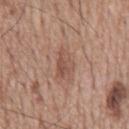The lesion was tiled from a total-body skin photograph and was not biopsied.
A male subject, about 60 years old.
The lesion is located on the back.
A roughly 15 mm field-of-view crop from a total-body skin photograph.